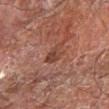biopsy status = catalogued during a skin exam; not biopsied
image = ~15 mm crop, total-body skin-cancer survey
anatomic site = the arm
lesion diameter = about 3 mm
subject = male, aged 68–72